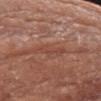| field | value |
|---|---|
| biopsy status | imaged on a skin check; not biopsied |
| illumination | white-light |
| acquisition | 15 mm crop, total-body photography |
| automated lesion analysis | an average lesion color of about L≈46 a*≈24 b*≈28 (CIELAB), about 6 CIELAB-L* units darker than the surrounding skin, and a normalized lesion–skin contrast near 4.5; a classifier nevus-likeness of about 0/100 and a detector confidence of about 85 out of 100 that the crop contains a lesion |
| lesion size | ≈3.5 mm |
| site | the right forearm |
| subject | female, approximately 75 years of age |